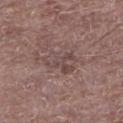Imaged during a routine full-body skin examination; the lesion was not biopsied and no histopathology is available.
Captured under white-light illumination.
The lesion is on the left lower leg.
A male subject, approximately 70 years of age.
A 15 mm close-up extracted from a 3D total-body photography capture.
Measured at roughly 4 mm in maximum diameter.